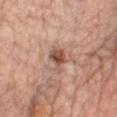biopsy status = imaged on a skin check; not biopsied
tile lighting = white-light illumination
image source = total-body-photography crop, ~15 mm field of view
size = ~3.5 mm (longest diameter)
site = the chest
subject = female, aged 53 to 57